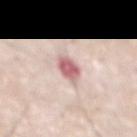Impression: Captured during whole-body skin photography for melanoma surveillance; the lesion was not biopsied. Clinical summary: A male subject aged approximately 80. The lesion is on the chest. Automated tile analysis of the lesion measured a mean CIELAB color near L≈64 a*≈25 b*≈20 and a normalized lesion–skin contrast near 9.5. The software also gave a border-irregularity rating of about 2/10 and internal color variation of about 3.5 on a 0–10 scale. It also reported a classifier nevus-likeness of about 0/100. A 15 mm crop from a total-body photograph taken for skin-cancer surveillance. The lesion's longest dimension is about 3 mm.